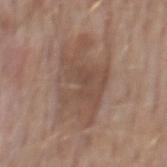workup = total-body-photography surveillance lesion; no biopsy | illumination = white-light | subject = male, in their mid-70s | site = the mid back | diameter = about 8 mm | image source = ~15 mm tile from a whole-body skin photo.